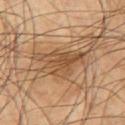<lesion>
<patient>
  <sex>male</sex>
  <age_approx>60</age_approx>
</patient>
<image>
  <source>total-body photography crop</source>
  <field_of_view_mm>15</field_of_view_mm>
</image>
<site>right upper arm</site>
</lesion>